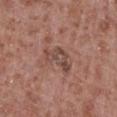biopsy status=total-body-photography surveillance lesion; no biopsy
image source=total-body-photography crop, ~15 mm field of view
site=the left lower leg
subject=male, aged approximately 55